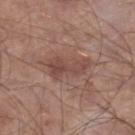Clinical impression:
Imaged during a routine full-body skin examination; the lesion was not biopsied and no histopathology is available.
Acquisition and patient details:
A close-up tile cropped from a whole-body skin photograph, about 15 mm across. A male patient, approximately 65 years of age. On the right lower leg. Imaged with white-light lighting.